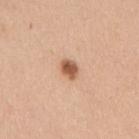| field | value |
|---|---|
| workup | total-body-photography surveillance lesion; no biopsy |
| tile lighting | white-light illumination |
| acquisition | ~15 mm crop, total-body skin-cancer survey |
| lesion size | about 2.5 mm |
| image-analysis metrics | a lesion area of about 4 mm², a shape eccentricity near 0.55, and a shape-asymmetry score of about 0.25 (0 = symmetric); an average lesion color of about L≈58 a*≈22 b*≈33 (CIELAB) and about 15 CIELAB-L* units darker than the surrounding skin; an automated nevus-likeness rating near 100 out of 100 and a detector confidence of about 100 out of 100 that the crop contains a lesion |
| subject | female, about 40 years old |
| location | the right upper arm |